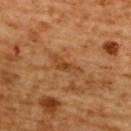The lesion was tiled from a total-body skin photograph and was not biopsied. The recorded lesion diameter is about 4 mm. The tile uses cross-polarized illumination. A female subject in their mid- to late 50s. A close-up tile cropped from a whole-body skin photograph, about 15 mm across. Located on the upper back.